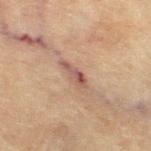Impression:
Imaged during a routine full-body skin examination; the lesion was not biopsied and no histopathology is available.
Image and clinical context:
On the right thigh. Cropped from a total-body skin-imaging series; the visible field is about 15 mm. Approximately 4 mm at its widest. This is a cross-polarized tile. A female patient, in their 80s.